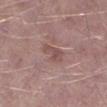<tbp_lesion>
  <biopsy_status>not biopsied; imaged during a skin examination</biopsy_status>
  <image>
    <source>total-body photography crop</source>
    <field_of_view_mm>15</field_of_view_mm>
  </image>
  <lesion_size>
    <long_diameter_mm_approx>3.0</long_diameter_mm_approx>
  </lesion_size>
  <lighting>white-light</lighting>
  <automated_metrics>
    <area_mm2_approx>5.0</area_mm2_approx>
    <eccentricity>0.8</eccentricity>
    <shape_asymmetry>0.35</shape_asymmetry>
  </automated_metrics>
  <site>left lower leg</site>
  <patient>
    <sex>male</sex>
    <age_approx>55</age_approx>
  </patient>
</tbp_lesion>